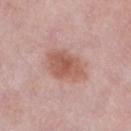Clinical impression:
Captured during whole-body skin photography for melanoma surveillance; the lesion was not biopsied.
Background:
The patient is a female aged 38 to 42. The lesion's longest dimension is about 5 mm. Located on the left lower leg. Captured under white-light illumination. A close-up tile cropped from a whole-body skin photograph, about 15 mm across.